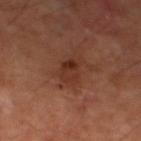Part of a total-body skin-imaging series; this lesion was reviewed on a skin check and was not flagged for biopsy. Measured at roughly 3.5 mm in maximum diameter. A male subject, in their 70s. Cropped from a total-body skin-imaging series; the visible field is about 15 mm. Imaged with cross-polarized lighting. The lesion is located on the left lower leg. An algorithmic analysis of the crop reported a border-irregularity index near 3/10.